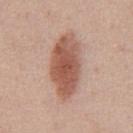follow-up — total-body-photography surveillance lesion; no biopsy | automated metrics — an area of roughly 22 mm², an outline eccentricity of about 0.85 (0 = round, 1 = elongated), and a symmetry-axis asymmetry near 0.15; border irregularity of about 2 on a 0–10 scale and a color-variation rating of about 4.5/10 | lighting — white-light | size — ≈7.5 mm | body site — the abdomen | image — ~15 mm crop, total-body skin-cancer survey | patient — male, approximately 40 years of age.